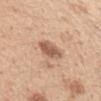<case>
  <biopsy_status>not biopsied; imaged during a skin examination</biopsy_status>
  <image>
    <source>total-body photography crop</source>
    <field_of_view_mm>15</field_of_view_mm>
  </image>
  <patient>
    <sex>female</sex>
    <age_approx>25</age_approx>
  </patient>
  <lesion_size>
    <long_diameter_mm_approx>3.0</long_diameter_mm_approx>
  </lesion_size>
  <lighting>white-light</lighting>
  <site>left upper arm</site>
  <automated_metrics>
    <cielab_L>58</cielab_L>
    <cielab_a>21</cielab_a>
    <cielab_b>31</cielab_b>
    <vs_skin_contrast_norm>8.0</vs_skin_contrast_norm>
  </automated_metrics>
</case>